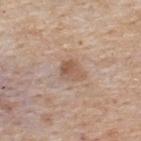Captured during whole-body skin photography for melanoma surveillance; the lesion was not biopsied. This is a white-light tile. The lesion is located on the upper back. This image is a 15 mm lesion crop taken from a total-body photograph. A male subject aged 53–57. The total-body-photography lesion software estimated a footprint of about 4 mm², an outline eccentricity of about 0.65 (0 = round, 1 = elongated), and a symmetry-axis asymmetry near 0.3. It also reported a border-irregularity index near 3/10, a color-variation rating of about 3.5/10, and peripheral color asymmetry of about 1.5. And it measured a classifier nevus-likeness of about 20/100. The lesion's longest dimension is about 2.5 mm.